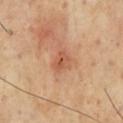No biopsy was performed on this lesion — it was imaged during a full skin examination and was not determined to be concerning.
A male subject roughly 70 years of age.
Measured at roughly 2.5 mm in maximum diameter.
From the chest.
A 15 mm close-up tile from a total-body photography series done for melanoma screening.
Automated tile analysis of the lesion measured a border-irregularity rating of about 2/10 and a peripheral color-asymmetry measure near 1.5.
Imaged with cross-polarized lighting.